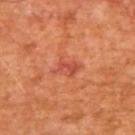Recorded during total-body skin imaging; not selected for excision or biopsy. Captured under cross-polarized illumination. A lesion tile, about 15 mm wide, cut from a 3D total-body photograph. On the upper back. A male subject in their mid- to late 60s. The lesion-visualizer software estimated a footprint of about 5 mm² and an eccentricity of roughly 0.8. The analysis additionally found a within-lesion color-variation index near 3/10. Longest diameter approximately 3.5 mm.